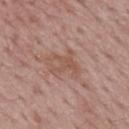Cropped from a whole-body photographic skin survey; the tile spans about 15 mm. The lesion is located on the mid back. A male subject aged 63 to 67. Imaged with white-light lighting. The lesion's longest dimension is about 3.5 mm. Automated tile analysis of the lesion measured a classifier nevus-likeness of about 0/100 and a lesion-detection confidence of about 95/100.